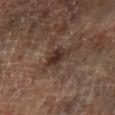Imaged during a routine full-body skin examination; the lesion was not biopsied and no histopathology is available.
Located on the left lower leg.
The subject is a male approximately 65 years of age.
A lesion tile, about 15 mm wide, cut from a 3D total-body photograph.
Imaged with cross-polarized lighting.
The total-body-photography lesion software estimated a lesion area of about 6 mm² and a shape eccentricity near 0.85.
Longest diameter approximately 3.5 mm.